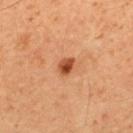{
  "biopsy_status": "not biopsied; imaged during a skin examination",
  "patient": {
    "sex": "male",
    "age_approx": 50
  },
  "site": "upper back",
  "automated_metrics": {
    "eccentricity": 0.65,
    "shape_asymmetry": 0.2,
    "lesion_detection_confidence_0_100": 100
  },
  "image": {
    "source": "total-body photography crop",
    "field_of_view_mm": 15
  }
}